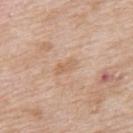| key | value |
|---|---|
| notes | total-body-photography surveillance lesion; no biopsy |
| imaging modality | ~15 mm tile from a whole-body skin photo |
| tile lighting | white-light |
| subject | male, in their mid-60s |
| body site | the upper back |
| lesion size | ~2.5 mm (longest diameter) |